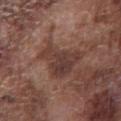subject = male, about 75 years old
acquisition = ~15 mm tile from a whole-body skin photo
location = the chest
automated metrics = a border-irregularity rating of about 6/10 and internal color variation of about 4 on a 0–10 scale; an automated nevus-likeness rating near 0 out of 100 and a detector confidence of about 90 out of 100 that the crop contains a lesion
diameter = about 5.5 mm
tile lighting = white-light illumination